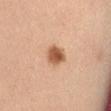Clinical impression: The lesion was tiled from a total-body skin photograph and was not biopsied.